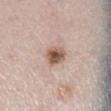  biopsy_status: not biopsied; imaged during a skin examination
  lighting: white-light
  lesion_size:
    long_diameter_mm_approx: 2.5
  image:
    source: total-body photography crop
    field_of_view_mm: 15
  site: leg
  patient:
    sex: female
    age_approx: 40